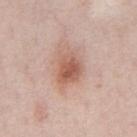biopsy_status: not biopsied; imaged during a skin examination
lighting: white-light
patient:
  sex: male
  age_approx: 50
lesion_size:
  long_diameter_mm_approx: 4.0
automated_metrics:
  cielab_L: 58
  cielab_a: 22
  cielab_b: 28
  vs_skin_darker_L: 11.0
  border_irregularity_0_10: 2.0
  color_variation_0_10: 5.5
  peripheral_color_asymmetry: 2.0
site: front of the torso
image:
  source: total-body photography crop
  field_of_view_mm: 15